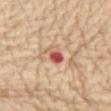This lesion was catalogued during total-body skin photography and was not selected for biopsy.
From the abdomen.
Cropped from a total-body skin-imaging series; the visible field is about 15 mm.
A female subject aged 68–72.